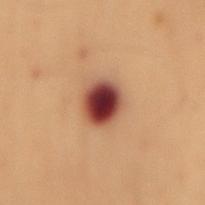Clinical impression: This lesion was catalogued during total-body skin photography and was not selected for biopsy. Context: The tile uses cross-polarized illumination. A female patient, in their 50s. A 15 mm close-up extracted from a 3D total-body photography capture. Automated tile analysis of the lesion measured a footprint of about 9.5 mm². The analysis additionally found a lesion color around L≈36 a*≈24 b*≈24 in CIELAB, about 19 CIELAB-L* units darker than the surrounding skin, and a normalized lesion–skin contrast near 16. The analysis additionally found a lesion-detection confidence of about 100/100. On the back. Measured at roughly 4 mm in maximum diameter.